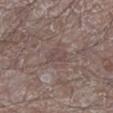Assessment: The lesion was photographed on a routine skin check and not biopsied; there is no pathology result. Acquisition and patient details: The lesion is on the left lower leg. A 15 mm close-up extracted from a 3D total-body photography capture. The recorded lesion diameter is about 2.5 mm. A male patient, approximately 65 years of age.